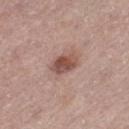image=~15 mm tile from a whole-body skin photo | automated metrics=a border-irregularity rating of about 1.5/10, a within-lesion color-variation index near 4.5/10, and peripheral color asymmetry of about 1.5 | patient=male, about 75 years old | location=the left thigh | lighting=white-light illumination | diameter=about 3 mm.